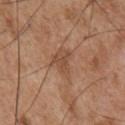Part of a total-body skin-imaging series; this lesion was reviewed on a skin check and was not flagged for biopsy. From the front of the torso. A male subject aged approximately 55. This is a white-light tile. The lesion's longest dimension is about 3.5 mm. A close-up tile cropped from a whole-body skin photograph, about 15 mm across.